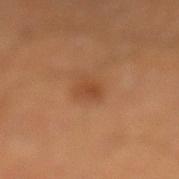{
  "biopsy_status": "not biopsied; imaged during a skin examination",
  "image": {
    "source": "total-body photography crop",
    "field_of_view_mm": 15
  },
  "site": "left lower leg",
  "patient": {
    "sex": "male",
    "age_approx": 40
  }
}